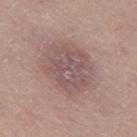| key | value |
|---|---|
| image | ~15 mm crop, total-body skin-cancer survey |
| size | ~6 mm (longest diameter) |
| automated metrics | a normalized border contrast of about 6; border irregularity of about 2 on a 0–10 scale, a within-lesion color-variation index near 3.5/10, and peripheral color asymmetry of about 1; a nevus-likeness score of about 0/100 and lesion-presence confidence of about 100/100 |
| body site | the left thigh |
| patient | female, approximately 50 years of age |